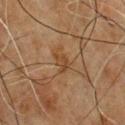Clinical impression: Imaged during a routine full-body skin examination; the lesion was not biopsied and no histopathology is available. Image and clinical context: The lesion is on the chest. The lesion's longest dimension is about 2.5 mm. Cropped from a total-body skin-imaging series; the visible field is about 15 mm. A male patient, aged 58–62. Captured under cross-polarized illumination.